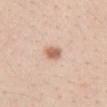{"image": {"source": "total-body photography crop", "field_of_view_mm": 15}, "site": "mid back", "lighting": "white-light", "patient": {"sex": "female", "age_approx": 25}}